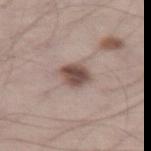| field | value |
|---|---|
| workup | imaged on a skin check; not biopsied |
| lighting | white-light illumination |
| diameter | about 3 mm |
| imaging modality | 15 mm crop, total-body photography |
| body site | the left thigh |
| automated metrics | a border-irregularity rating of about 1.5/10, a color-variation rating of about 4/10, and peripheral color asymmetry of about 1.5; an automated nevus-likeness rating near 85 out of 100 and lesion-presence confidence of about 100/100 |
| patient | male, aged 68 to 72 |